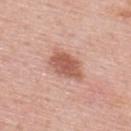Part of a total-body skin-imaging series; this lesion was reviewed on a skin check and was not flagged for biopsy. The subject is a male aged 53 to 57. Captured under white-light illumination. A 15 mm crop from a total-body photograph taken for skin-cancer surveillance. On the upper back.